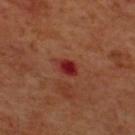Imaged during a routine full-body skin examination; the lesion was not biopsied and no histopathology is available.
The recorded lesion diameter is about 3 mm.
On the upper back.
The subject is a male about 70 years old.
Automated image analysis of the tile measured a shape eccentricity near 0.7 and two-axis asymmetry of about 0.25. The software also gave a mean CIELAB color near L≈30 a*≈33 b*≈27, roughly 10 lightness units darker than nearby skin, and a lesion-to-skin contrast of about 10 (normalized; higher = more distinct). The analysis additionally found a border-irregularity index near 2/10 and internal color variation of about 3 on a 0–10 scale.
This is a cross-polarized tile.
A 15 mm close-up tile from a total-body photography series done for melanoma screening.